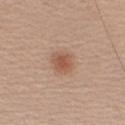follow-up = imaged on a skin check; not biopsied | size = about 2.5 mm | subject = male, aged 58–62 | image = ~15 mm tile from a whole-body skin photo | lighting = white-light illumination | anatomic site = the right upper arm.